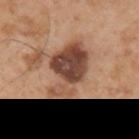Case summary:
• follow-up — imaged on a skin check; not biopsied
• body site — the left upper arm
• subject — male, in their mid-50s
• size — about 7.5 mm
• image source — 15 mm crop, total-body photography